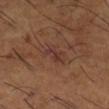Clinical impression: The lesion was tiled from a total-body skin photograph and was not biopsied. Context: The total-body-photography lesion software estimated an outline eccentricity of about 0.9 (0 = round, 1 = elongated) and a symmetry-axis asymmetry near 0.55. It also reported a lesion color around L≈34 a*≈21 b*≈24 in CIELAB and a lesion-to-skin contrast of about 6.5 (normalized; higher = more distinct). It also reported a border-irregularity index near 5.5/10, internal color variation of about 0 on a 0–10 scale, and radial color variation of about 0. The software also gave an automated nevus-likeness rating near 0 out of 100 and lesion-presence confidence of about 100/100. Captured under cross-polarized illumination. A lesion tile, about 15 mm wide, cut from a 3D total-body photograph. From the leg. A male subject, about 65 years old.